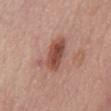follow-up: no biopsy performed (imaged during a skin exam)
size: about 5 mm
lighting: white-light illumination
acquisition: ~15 mm crop, total-body skin-cancer survey
patient: female, aged 63 to 67
site: the abdomen